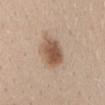This is a white-light tile. The subject is a female aged around 30. A close-up tile cropped from a whole-body skin photograph, about 15 mm across. About 4 mm across. From the mid back.